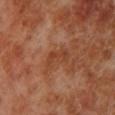Assessment:
This lesion was catalogued during total-body skin photography and was not selected for biopsy.
Acquisition and patient details:
Imaged with cross-polarized lighting. The lesion is on the leg. Cropped from a total-body skin-imaging series; the visible field is about 15 mm. Measured at roughly 3.5 mm in maximum diameter. A male subject, about 70 years old.